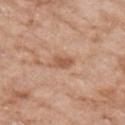Assessment: Captured during whole-body skin photography for melanoma surveillance; the lesion was not biopsied. Background: A region of skin cropped from a whole-body photographic capture, roughly 15 mm wide. The recorded lesion diameter is about 3 mm. The tile uses white-light illumination. The lesion-visualizer software estimated a lesion area of about 4 mm² and an outline eccentricity of about 0.8 (0 = round, 1 = elongated). The analysis additionally found a border-irregularity rating of about 3.5/10 and a peripheral color-asymmetry measure near 0.5. The analysis additionally found a nevus-likeness score of about 20/100. A female subject, approximately 75 years of age. Located on the right upper arm.